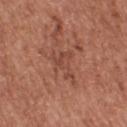Impression: No biopsy was performed on this lesion — it was imaged during a full skin examination and was not determined to be concerning. Context: A male patient about 45 years old. A roughly 15 mm field-of-view crop from a total-body skin photograph. Located on the chest.